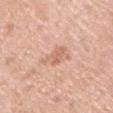| key | value |
|---|---|
| notes | catalogued during a skin exam; not biopsied |
| illumination | white-light illumination |
| body site | the front of the torso |
| patient | male, aged 43–47 |
| TBP lesion metrics | a lesion color around L≈65 a*≈22 b*≈31 in CIELAB, roughly 8 lightness units darker than nearby skin, and a normalized lesion–skin contrast near 5.5; a border-irregularity index near 4.5/10, a within-lesion color-variation index near 2/10, and a peripheral color-asymmetry measure near 0.5 |
| imaging modality | ~15 mm tile from a whole-body skin photo |
| size | about 4 mm |